Cropped from a whole-body photographic skin survey; the tile spans about 15 mm · a male subject in their mid- to late 40s · located on the left forearm: 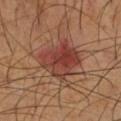The lesion-visualizer software estimated a border-irregularity index near 3/10, a color-variation rating of about 6/10, and radial color variation of about 2.
Approximately 5 mm at its widest.
Captured under cross-polarized illumination.
Histopathological examination showed a squamous cell carcinoma in situ.